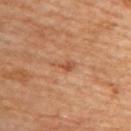Captured during whole-body skin photography for melanoma surveillance; the lesion was not biopsied. Located on the upper back. A 15 mm close-up extracted from a 3D total-body photography capture. A female subject, about 65 years old. Approximately 2.5 mm at its widest.